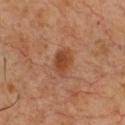Q: Is there a histopathology result?
A: total-body-photography surveillance lesion; no biopsy
Q: What is the anatomic site?
A: the chest
Q: Who is the patient?
A: male, aged 48–52
Q: How large is the lesion?
A: about 3.5 mm
Q: How was this image acquired?
A: 15 mm crop, total-body photography